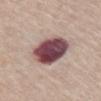Recorded during total-body skin imaging; not selected for excision or biopsy. A roughly 15 mm field-of-view crop from a total-body skin photograph. A female patient, in their 70s. Imaged with white-light lighting. The recorded lesion diameter is about 5 mm. Located on the front of the torso.